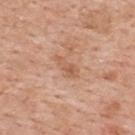{
  "biopsy_status": "not biopsied; imaged during a skin examination",
  "lighting": "white-light",
  "site": "upper back",
  "lesion_size": {
    "long_diameter_mm_approx": 3.5
  },
  "image": {
    "source": "total-body photography crop",
    "field_of_view_mm": 15
  },
  "automated_metrics": {
    "shape_asymmetry": 0.35,
    "nevus_likeness_0_100": 0
  },
  "patient": {
    "sex": "female",
    "age_approx": 40
  }
}